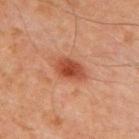biopsy status=catalogued during a skin exam; not biopsied
imaging modality=~15 mm tile from a whole-body skin photo
illumination=cross-polarized illumination
image-analysis metrics=lesion-presence confidence of about 100/100
site=the chest
patient=male, about 70 years old